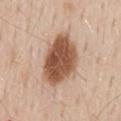{
  "biopsy_status": "not biopsied; imaged during a skin examination",
  "site": "mid back",
  "lesion_size": {
    "long_diameter_mm_approx": 7.0
  },
  "patient": {
    "sex": "male",
    "age_approx": 60
  },
  "lighting": "white-light",
  "automated_metrics": {
    "area_mm2_approx": 22.0,
    "eccentricity": 0.75,
    "shape_asymmetry": 0.2,
    "cielab_L": 53,
    "cielab_a": 21,
    "cielab_b": 31,
    "vs_skin_darker_L": 18.0,
    "color_variation_0_10": 5.5
  },
  "image": {
    "source": "total-body photography crop",
    "field_of_view_mm": 15
  }
}